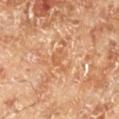Impression:
The lesion was photographed on a routine skin check and not biopsied; there is no pathology result.
Image and clinical context:
The subject is a male aged 68 to 72. Cropped from a whole-body photographic skin survey; the tile spans about 15 mm. Captured under cross-polarized illumination. Located on the leg. The total-body-photography lesion software estimated border irregularity of about 6 on a 0–10 scale, a within-lesion color-variation index near 3/10, and peripheral color asymmetry of about 1. The analysis additionally found a classifier nevus-likeness of about 0/100 and a lesion-detection confidence of about 65/100. The lesion's longest dimension is about 3 mm.